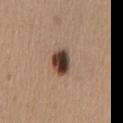<record>
  <biopsy_status>not biopsied; imaged during a skin examination</biopsy_status>
  <site>front of the torso</site>
  <automated_metrics>
    <border_irregularity_0_10>2.0</border_irregularity_0_10>
    <color_variation_0_10>9.0</color_variation_0_10>
    <peripheral_color_asymmetry>3.0</peripheral_color_asymmetry>
    <lesion_detection_confidence_0_100>100</lesion_detection_confidence_0_100>
  </automated_metrics>
  <lesion_size>
    <long_diameter_mm_approx>3.5</long_diameter_mm_approx>
  </lesion_size>
  <image>
    <source>total-body photography crop</source>
    <field_of_view_mm>15</field_of_view_mm>
  </image>
  <patient>
    <sex>female</sex>
    <age_approx>60</age_approx>
  </patient>
  <lighting>white-light</lighting>
</record>